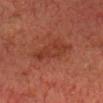biopsy status=total-body-photography surveillance lesion; no biopsy
lighting=cross-polarized
image source=total-body-photography crop, ~15 mm field of view
subject=male, roughly 75 years of age
automated lesion analysis=an eccentricity of roughly 0.9 and a symmetry-axis asymmetry near 0.25; a mean CIELAB color near L≈31 a*≈24 b*≈27 and roughly 6 lightness units darker than nearby skin; a peripheral color-asymmetry measure near 1; lesion-presence confidence of about 100/100
lesion diameter=~5 mm (longest diameter)
site=the head or neck